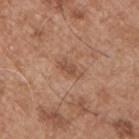biopsy status: total-body-photography surveillance lesion; no biopsy | patient: male, approximately 55 years of age | site: the chest | imaging modality: ~15 mm crop, total-body skin-cancer survey.